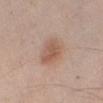<lesion>
<biopsy_status>not biopsied; imaged during a skin examination</biopsy_status>
<site>right forearm</site>
<lesion_size>
  <long_diameter_mm_approx>3.5</long_diameter_mm_approx>
</lesion_size>
<image>
  <source>total-body photography crop</source>
  <field_of_view_mm>15</field_of_view_mm>
</image>
<lighting>white-light</lighting>
<patient>
  <sex>male</sex>
  <age_approx>45</age_approx>
</patient>
</lesion>